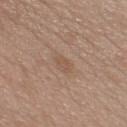Captured during whole-body skin photography for melanoma surveillance; the lesion was not biopsied. On the chest. A male patient, about 40 years old. The tile uses white-light illumination. Measured at roughly 2.5 mm in maximum diameter. Cropped from a total-body skin-imaging series; the visible field is about 15 mm.